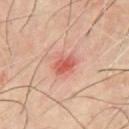Imaged during a routine full-body skin examination; the lesion was not biopsied and no histopathology is available. A roughly 15 mm field-of-view crop from a total-body skin photograph. A male subject, in their mid- to late 60s. Automated image analysis of the tile measured a mean CIELAB color near L≈58 a*≈33 b*≈31. The analysis additionally found a border-irregularity index near 2/10, a within-lesion color-variation index near 4.5/10, and peripheral color asymmetry of about 1.5. Longest diameter approximately 2.5 mm. The lesion is located on the front of the torso.